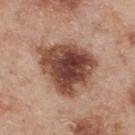notes = no biopsy performed (imaged during a skin exam)
tile lighting = white-light illumination
site = the upper back
patient = male, roughly 55 years of age
imaging modality = ~15 mm crop, total-body skin-cancer survey